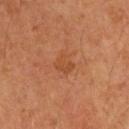biopsy status: no biopsy performed (imaged during a skin exam) | imaging modality: total-body-photography crop, ~15 mm field of view | lighting: cross-polarized illumination | body site: the arm | diameter: ≈2.5 mm | patient: male, approximately 60 years of age.